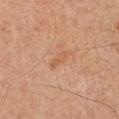This lesion was catalogued during total-body skin photography and was not selected for biopsy.
A 15 mm crop from a total-body photograph taken for skin-cancer surveillance.
The tile uses cross-polarized illumination.
Located on the left lower leg.
Measured at roughly 3.5 mm in maximum diameter.
A male subject, aged around 50.